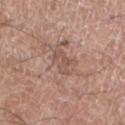Part of a total-body skin-imaging series; this lesion was reviewed on a skin check and was not flagged for biopsy.
A male patient, aged around 60.
An algorithmic analysis of the crop reported a lesion area of about 3.5 mm², an eccentricity of roughly 0.7, and a shape-asymmetry score of about 0.5 (0 = symmetric). It also reported a mean CIELAB color near L≈52 a*≈19 b*≈26, roughly 7 lightness units darker than nearby skin, and a normalized border contrast of about 5. The software also gave border irregularity of about 7.5 on a 0–10 scale, internal color variation of about 0 on a 0–10 scale, and peripheral color asymmetry of about 0.
The tile uses white-light illumination.
The lesion's longest dimension is about 2.5 mm.
From the left lower leg.
A roughly 15 mm field-of-view crop from a total-body skin photograph.